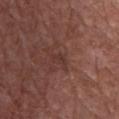- workup — imaged on a skin check; not biopsied
- acquisition — 15 mm crop, total-body photography
- patient — male, approximately 75 years of age
- body site — the chest
- tile lighting — white-light illumination
- lesion size — about 2.5 mm